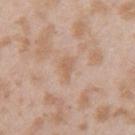The lesion was photographed on a routine skin check and not biopsied; there is no pathology result. A female subject, in their mid-20s. This image is a 15 mm lesion crop taken from a total-body photograph. Captured under white-light illumination. The lesion is located on the left upper arm. About 2.5 mm across.